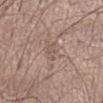The lesion was tiled from a total-body skin photograph and was not biopsied.
A 15 mm close-up extracted from a 3D total-body photography capture.
From the chest.
The subject is a male about 70 years old.